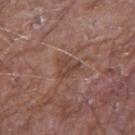site: the arm | imaging modality: 15 mm crop, total-body photography | subject: male, aged around 65 | tile lighting: white-light illumination | image-analysis metrics: a footprint of about 4 mm², an eccentricity of roughly 0.55, and two-axis asymmetry of about 0.55; a border-irregularity index near 5/10, a within-lesion color-variation index near 2/10, and peripheral color asymmetry of about 0.5 | lesion size: ~2.5 mm (longest diameter).